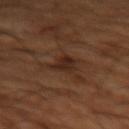Impression: Part of a total-body skin-imaging series; this lesion was reviewed on a skin check and was not flagged for biopsy. Image and clinical context: Located on the left thigh. Longest diameter approximately 3 mm. The patient is a male in their mid-60s. Automated image analysis of the tile measured an area of roughly 6 mm² and an outline eccentricity of about 0.6 (0 = round, 1 = elongated). The software also gave an average lesion color of about L≈26 a*≈18 b*≈25 (CIELAB). Imaged with cross-polarized lighting. A lesion tile, about 15 mm wide, cut from a 3D total-body photograph.